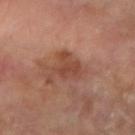No biopsy was performed on this lesion — it was imaged during a full skin examination and was not determined to be concerning.
A female patient in their mid-60s.
The lesion is on the right forearm.
A 15 mm close-up extracted from a 3D total-body photography capture.